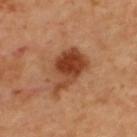The lesion is located on the upper back. Cropped from a total-body skin-imaging series; the visible field is about 15 mm. A male subject, aged 58–62. Captured under cross-polarized illumination.